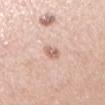Assessment: This lesion was catalogued during total-body skin photography and was not selected for biopsy. Context: A 15 mm crop from a total-body photograph taken for skin-cancer surveillance. Imaged with white-light lighting. Located on the right upper arm. The subject is a female in their 60s.